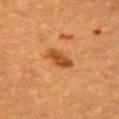Acquisition and patient details: The recorded lesion diameter is about 3.5 mm. Cropped from a total-body skin-imaging series; the visible field is about 15 mm. The lesion-visualizer software estimated an area of roughly 5.5 mm² and a shape-asymmetry score of about 0.2 (0 = symmetric). The analysis additionally found a lesion color around L≈42 a*≈24 b*≈38 in CIELAB, a lesion–skin lightness drop of about 11, and a normalized lesion–skin contrast near 8.5. The software also gave border irregularity of about 2 on a 0–10 scale, internal color variation of about 3 on a 0–10 scale, and a peripheral color-asymmetry measure near 1. The analysis additionally found a classifier nevus-likeness of about 95/100 and a detector confidence of about 100 out of 100 that the crop contains a lesion. From the chest. A female subject aged 53–57.